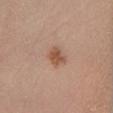biopsy_status: not biopsied; imaged during a skin examination
image:
  source: total-body photography crop
  field_of_view_mm: 15
automated_metrics:
  area_mm2_approx: 4.0
  shape_asymmetry: 0.25
  nevus_likeness_0_100: 90
site: right lower leg
lighting: cross-polarized
patient:
  sex: male
  age_approx: 50
lesion_size:
  long_diameter_mm_approx: 2.5